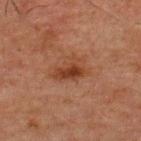This lesion was catalogued during total-body skin photography and was not selected for biopsy. A male patient aged 48–52. The recorded lesion diameter is about 4 mm. The lesion is on the upper back. This image is a 15 mm lesion crop taken from a total-body photograph. An algorithmic analysis of the crop reported a lesion area of about 9 mm², an eccentricity of roughly 0.8, and a shape-asymmetry score of about 0.3 (0 = symmetric). The software also gave a border-irregularity index near 3.5/10. It also reported a nevus-likeness score of about 65/100 and a detector confidence of about 100 out of 100 that the crop contains a lesion.